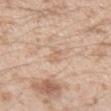This is a white-light tile.
The recorded lesion diameter is about 2.5 mm.
Located on the left forearm.
A male subject, approximately 25 years of age.
A roughly 15 mm field-of-view crop from a total-body skin photograph.
The lesion-visualizer software estimated an area of roughly 3 mm², an eccentricity of roughly 0.9, and two-axis asymmetry of about 0.3. It also reported an average lesion color of about L≈65 a*≈17 b*≈32 (CIELAB), about 7 CIELAB-L* units darker than the surrounding skin, and a normalized lesion–skin contrast near 4.5.